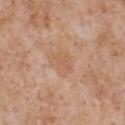{
  "biopsy_status": "not biopsied; imaged during a skin examination",
  "lesion_size": {
    "long_diameter_mm_approx": 3.0
  },
  "patient": {
    "sex": "male",
    "age_approx": 65
  },
  "automated_metrics": {
    "area_mm2_approx": 5.5,
    "eccentricity": 0.55,
    "cielab_L": 59,
    "cielab_a": 20,
    "cielab_b": 33,
    "vs_skin_contrast_norm": 4.5,
    "border_irregularity_0_10": 3.0,
    "color_variation_0_10": 2.0,
    "peripheral_color_asymmetry": 0.5,
    "nevus_likeness_0_100": 0
  },
  "lighting": "white-light",
  "image": {
    "source": "total-body photography crop",
    "field_of_view_mm": 15
  },
  "site": "chest"
}